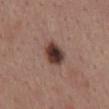Impression:
Imaged during a routine full-body skin examination; the lesion was not biopsied and no histopathology is available.
Acquisition and patient details:
Captured under white-light illumination. Cropped from a total-body skin-imaging series; the visible field is about 15 mm. On the mid back. The patient is a female aged 48–52. Measured at roughly 3.5 mm in maximum diameter.